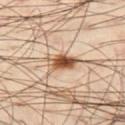| field | value |
|---|---|
| notes | imaged on a skin check; not biopsied |
| image-analysis metrics | a footprint of about 6 mm², an outline eccentricity of about 0.8 (0 = round, 1 = elongated), and a symmetry-axis asymmetry near 0.25; a border-irregularity index near 2.5/10, a color-variation rating of about 6.5/10, and peripheral color asymmetry of about 2; a nevus-likeness score of about 100/100 and lesion-presence confidence of about 100/100 |
| patient | male, aged approximately 50 |
| anatomic site | the leg |
| lesion diameter | ~3.5 mm (longest diameter) |
| lighting | cross-polarized illumination |
| image | ~15 mm tile from a whole-body skin photo |